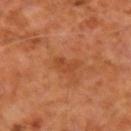Part of a total-body skin-imaging series; this lesion was reviewed on a skin check and was not flagged for biopsy. The subject is a male aged 58 to 62. Captured under cross-polarized illumination. An algorithmic analysis of the crop reported a lesion area of about 3 mm², a shape eccentricity near 0.8, and two-axis asymmetry of about 0.5. The software also gave a mean CIELAB color near L≈43 a*≈27 b*≈37. And it measured a border-irregularity index near 5/10 and a peripheral color-asymmetry measure near 0. The software also gave an automated nevus-likeness rating near 0 out of 100. The lesion's longest dimension is about 2.5 mm. Cropped from a whole-body photographic skin survey; the tile spans about 15 mm. The lesion is on the left forearm.